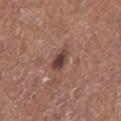Clinical impression: This lesion was catalogued during total-body skin photography and was not selected for biopsy. Context: The lesion-visualizer software estimated a lesion area of about 4 mm² and a symmetry-axis asymmetry near 0.3. The software also gave a lesion–skin lightness drop of about 13 and a normalized border contrast of about 10. The software also gave border irregularity of about 2.5 on a 0–10 scale, a color-variation rating of about 3.5/10, and a peripheral color-asymmetry measure near 1. From the leg. The subject is a male aged 73 to 77. About 3 mm across. This is a white-light tile. A 15 mm close-up extracted from a 3D total-body photography capture.